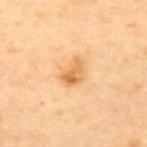| field | value |
|---|---|
| biopsy status | total-body-photography surveillance lesion; no biopsy |
| lesion size | about 3 mm |
| patient | male, aged around 65 |
| anatomic site | the back |
| imaging modality | ~15 mm tile from a whole-body skin photo |
| lighting | cross-polarized |
| image-analysis metrics | a footprint of about 5 mm², a shape eccentricity near 0.75, and a shape-asymmetry score of about 0.4 (0 = symmetric) |